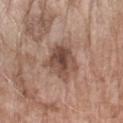biopsy status: catalogued during a skin exam; not biopsied | automated metrics: a footprint of about 13 mm² and a shape-asymmetry score of about 0.25 (0 = symmetric); a lesion–skin lightness drop of about 12 and a normalized border contrast of about 8.5; a border-irregularity rating of about 3/10, internal color variation of about 6.5 on a 0–10 scale, and peripheral color asymmetry of about 2.5; an automated nevus-likeness rating near 5 out of 100 and a lesion-detection confidence of about 100/100 | patient: female, in their mid-70s | illumination: white-light | site: the arm | lesion size: about 4.5 mm | acquisition: ~15 mm tile from a whole-body skin photo.